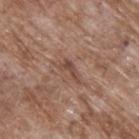Assessment: The lesion was tiled from a total-body skin photograph and was not biopsied. Background: Approximately 2.5 mm at its widest. Cropped from a whole-body photographic skin survey; the tile spans about 15 mm. On the upper back. A male subject about 70 years old.